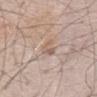Assessment: Captured during whole-body skin photography for melanoma surveillance; the lesion was not biopsied. Acquisition and patient details: A close-up tile cropped from a whole-body skin photograph, about 15 mm across. From the chest. The subject is a male aged around 70. Captured under white-light illumination. Measured at roughly 3 mm in maximum diameter.